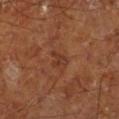The lesion was photographed on a routine skin check and not biopsied; there is no pathology result. A male patient aged approximately 65. Located on the right lower leg. A lesion tile, about 15 mm wide, cut from a 3D total-body photograph.